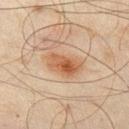workup: total-body-photography surveillance lesion; no biopsy | illumination: cross-polarized illumination | anatomic site: the left thigh | patient: male, aged 43–47 | image: ~15 mm crop, total-body skin-cancer survey | size: ≈4.5 mm.